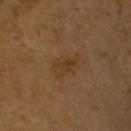Recorded during total-body skin imaging; not selected for excision or biopsy.
This image is a 15 mm lesion crop taken from a total-body photograph.
The lesion-visualizer software estimated a footprint of about 6 mm², an outline eccentricity of about 0.8 (0 = round, 1 = elongated), and a symmetry-axis asymmetry near 0.35. The software also gave a mean CIELAB color near L≈36 a*≈17 b*≈32, about 5 CIELAB-L* units darker than the surrounding skin, and a normalized border contrast of about 5.5. The analysis additionally found border irregularity of about 4 on a 0–10 scale, internal color variation of about 2.5 on a 0–10 scale, and radial color variation of about 0.5.
A male subject aged around 65.
Longest diameter approximately 3.5 mm.
The lesion is located on the arm.